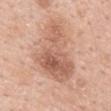{"biopsy_status": "not biopsied; imaged during a skin examination", "patient": {"sex": "male", "age_approx": 55}, "lighting": "white-light", "image": {"source": "total-body photography crop", "field_of_view_mm": 15}, "site": "back"}